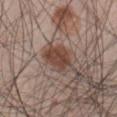Part of a total-body skin-imaging series; this lesion was reviewed on a skin check and was not flagged for biopsy.
A male patient, about 45 years old.
An algorithmic analysis of the crop reported an area of roughly 9 mm², an outline eccentricity of about 0.65 (0 = round, 1 = elongated), and two-axis asymmetry of about 0.2. And it measured roughly 12 lightness units darker than nearby skin and a normalized lesion–skin contrast near 9.5. The software also gave a nevus-likeness score of about 95/100 and a detector confidence of about 100 out of 100 that the crop contains a lesion.
On the front of the torso.
A 15 mm close-up tile from a total-body photography series done for melanoma screening.
Longest diameter approximately 4 mm.